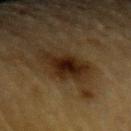notes: imaged on a skin check; not biopsied
site: the left upper arm
image: total-body-photography crop, ~15 mm field of view
illumination: cross-polarized
patient: male, roughly 85 years of age or older